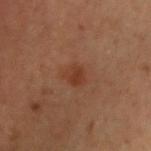Recorded during total-body skin imaging; not selected for excision or biopsy.
The total-body-photography lesion software estimated an area of roughly 4.5 mm², a shape eccentricity near 0.55, and a symmetry-axis asymmetry near 0.25. And it measured a lesion–skin lightness drop of about 6. It also reported a classifier nevus-likeness of about 80/100 and a lesion-detection confidence of about 100/100.
From the arm.
This image is a 15 mm lesion crop taken from a total-body photograph.
A male subject about 50 years old.